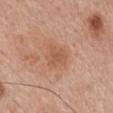notes = total-body-photography surveillance lesion; no biopsy | patient = male, aged 53–57 | illumination = white-light illumination | lesion size = about 3 mm | location = the back | image = ~15 mm crop, total-body skin-cancer survey.